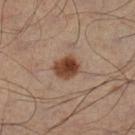Q: Was a biopsy performed?
A: imaged on a skin check; not biopsied
Q: What is the lesion's diameter?
A: ≈3 mm
Q: Illumination type?
A: cross-polarized illumination
Q: Where on the body is the lesion?
A: the leg
Q: How was this image acquired?
A: 15 mm crop, total-body photography
Q: Patient demographics?
A: male, aged 48–52